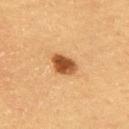Assessment:
No biopsy was performed on this lesion — it was imaged during a full skin examination and was not determined to be concerning.
Image and clinical context:
A male patient, approximately 60 years of age. A close-up tile cropped from a whole-body skin photograph, about 15 mm across. Captured under cross-polarized illumination. On the upper back. Longest diameter approximately 3.5 mm. The lesion-visualizer software estimated a footprint of about 6 mm² and an eccentricity of roughly 0.7. The software also gave an average lesion color of about L≈46 a*≈23 b*≈38 (CIELAB), about 17 CIELAB-L* units darker than the surrounding skin, and a lesion-to-skin contrast of about 11.5 (normalized; higher = more distinct).